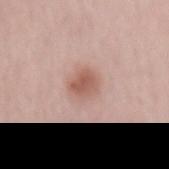notes: total-body-photography surveillance lesion; no biopsy | location: the lower back | imaging modality: ~15 mm tile from a whole-body skin photo | lesion diameter: ~3 mm (longest diameter) | subject: male, about 65 years old | TBP lesion metrics: an area of roughly 6 mm², an eccentricity of roughly 0.5, and two-axis asymmetry of about 0.15; an average lesion color of about L≈57 a*≈22 b*≈26 (CIELAB), roughly 11 lightness units darker than nearby skin, and a lesion-to-skin contrast of about 7.5 (normalized; higher = more distinct); a nevus-likeness score of about 95/100 and a lesion-detection confidence of about 100/100.